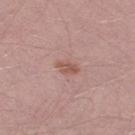Notes:
* acquisition — 15 mm crop, total-body photography
* diameter — about 2.5 mm
* illumination — white-light
* patient — male, aged 28 to 32